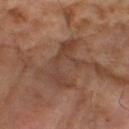The lesion was photographed on a routine skin check and not biopsied; there is no pathology result. Cropped from a whole-body photographic skin survey; the tile spans about 15 mm. The subject is a male aged approximately 65. About 6 mm across. The tile uses cross-polarized illumination.